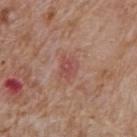Q: Was a biopsy performed?
A: no biopsy performed (imaged during a skin exam)
Q: Who is the patient?
A: male, approximately 60 years of age
Q: Lesion location?
A: the mid back
Q: What kind of image is this?
A: ~15 mm tile from a whole-body skin photo